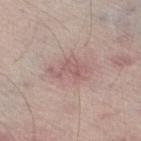{
  "biopsy_status": "not biopsied; imaged during a skin examination",
  "site": "left lower leg",
  "image": {
    "source": "total-body photography crop",
    "field_of_view_mm": 15
  },
  "patient": {
    "sex": "male",
    "age_approx": 65
  },
  "automated_metrics": {
    "area_mm2_approx": 5.5,
    "eccentricity": 0.9,
    "shape_asymmetry": 0.5,
    "cielab_L": 57,
    "cielab_a": 20,
    "cielab_b": 20,
    "vs_skin_darker_L": 8.0,
    "vs_skin_contrast_norm": 5.5,
    "border_irregularity_0_10": 7.0,
    "color_variation_0_10": 2.0,
    "peripheral_color_asymmetry": 0.5,
    "nevus_likeness_0_100": 0,
    "lesion_detection_confidence_0_100": 100
  }
}